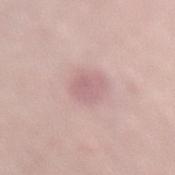<lesion>
  <biopsy_status>not biopsied; imaged during a skin examination</biopsy_status>
  <site>back</site>
  <lighting>white-light</lighting>
  <image>
    <source>total-body photography crop</source>
    <field_of_view_mm>15</field_of_view_mm>
  </image>
  <lesion_size>
    <long_diameter_mm_approx>3.0</long_diameter_mm_approx>
  </lesion_size>
  <patient>
    <sex>female</sex>
    <age_approx>40</age_approx>
  </patient>
  <automated_metrics>
    <nevus_likeness_0_100>5</nevus_likeness_0_100>
    <lesion_detection_confidence_0_100>100</lesion_detection_confidence_0_100>
  </automated_metrics>
</lesion>